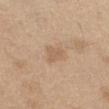Clinical impression:
Part of a total-body skin-imaging series; this lesion was reviewed on a skin check and was not flagged for biopsy.
Image and clinical context:
The lesion is located on the abdomen. A region of skin cropped from a whole-body photographic capture, roughly 15 mm wide. The patient is a male about 70 years old. Automated tile analysis of the lesion measured a lesion area of about 3.5 mm², an eccentricity of roughly 0.7, and a symmetry-axis asymmetry near 0.3. It also reported internal color variation of about 1 on a 0–10 scale and peripheral color asymmetry of about 0.5. The software also gave lesion-presence confidence of about 100/100.